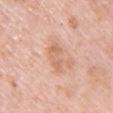No biopsy was performed on this lesion — it was imaged during a full skin examination and was not determined to be concerning. A roughly 15 mm field-of-view crop from a total-body skin photograph. The tile uses white-light illumination. Located on the chest. A female subject, aged 63 to 67. The lesion's longest dimension is about 4 mm.